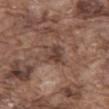Part of a total-body skin-imaging series; this lesion was reviewed on a skin check and was not flagged for biopsy. About 3.5 mm across. A 15 mm close-up extracted from a 3D total-body photography capture. On the abdomen. The total-body-photography lesion software estimated a lesion area of about 6.5 mm², a shape eccentricity near 0.7, and a shape-asymmetry score of about 0.35 (0 = symmetric). The software also gave a lesion color around L≈41 a*≈18 b*≈24 in CIELAB, roughly 9 lightness units darker than nearby skin, and a normalized border contrast of about 7. It also reported a border-irregularity rating of about 4/10 and internal color variation of about 4.5 on a 0–10 scale. A male patient approximately 75 years of age.